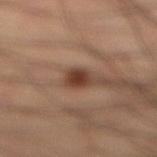follow-up — catalogued during a skin exam; not biopsied | image source — 15 mm crop, total-body photography | tile lighting — cross-polarized | automated metrics — a lesion area of about 4 mm², an outline eccentricity of about 0.7 (0 = round, 1 = elongated), and a symmetry-axis asymmetry near 0.25; a lesion–skin lightness drop of about 13 and a normalized border contrast of about 11 | subject — male, aged around 65 | site — the right lower leg | size — ~2.5 mm (longest diameter).